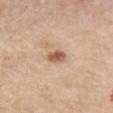Recorded during total-body skin imaging; not selected for excision or biopsy. The patient is a female about 70 years old. The recorded lesion diameter is about 2.5 mm. Located on the chest. A close-up tile cropped from a whole-body skin photograph, about 15 mm across. The tile uses white-light illumination.